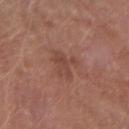A male patient, about 55 years old.
Automated tile analysis of the lesion measured an eccentricity of roughly 0.6. It also reported roughly 6 lightness units darker than nearby skin and a normalized lesion–skin contrast near 5. The analysis additionally found a color-variation rating of about 2.5/10 and radial color variation of about 1. It also reported an automated nevus-likeness rating near 0 out of 100 and lesion-presence confidence of about 100/100.
Cropped from a total-body skin-imaging series; the visible field is about 15 mm.
From the left forearm.